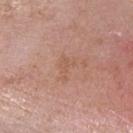Captured during whole-body skin photography for melanoma surveillance; the lesion was not biopsied. Longest diameter approximately 3 mm. The total-body-photography lesion software estimated a lesion area of about 4 mm² and a shape eccentricity near 0.8. The analysis additionally found lesion-presence confidence of about 100/100. Imaged with white-light lighting. A 15 mm crop from a total-body photograph taken for skin-cancer surveillance. Located on the head or neck. A female subject, aged 38–42.